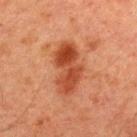No biopsy was performed on this lesion — it was imaged during a full skin examination and was not determined to be concerning. Imaged with cross-polarized lighting. The subject is a male aged approximately 60. On the upper back. Longest diameter approximately 6 mm. A roughly 15 mm field-of-view crop from a total-body skin photograph. Automated tile analysis of the lesion measured an outline eccentricity of about 0.9 (0 = round, 1 = elongated) and two-axis asymmetry of about 0.25. The software also gave a mean CIELAB color near L≈37 a*≈26 b*≈31 and a normalized border contrast of about 9. And it measured border irregularity of about 3.5 on a 0–10 scale, internal color variation of about 5 on a 0–10 scale, and peripheral color asymmetry of about 2. It also reported a nevus-likeness score of about 75/100 and lesion-presence confidence of about 100/100.